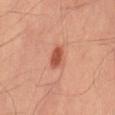Clinical impression:
Part of a total-body skin-imaging series; this lesion was reviewed on a skin check and was not flagged for biopsy.
Background:
Imaged with cross-polarized lighting. The patient is a male about 70 years old. The lesion is located on the right thigh. A roughly 15 mm field-of-view crop from a total-body skin photograph.